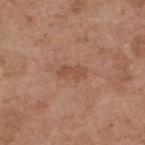Q: Was a biopsy performed?
A: no biopsy performed (imaged during a skin exam)
Q: What kind of image is this?
A: ~15 mm crop, total-body skin-cancer survey
Q: Where on the body is the lesion?
A: the upper back
Q: Patient demographics?
A: male, about 55 years old
Q: Lesion size?
A: ~3 mm (longest diameter)
Q: What did automated image analysis measure?
A: an eccentricity of roughly 0.9 and a shape-asymmetry score of about 0.3 (0 = symmetric); border irregularity of about 3.5 on a 0–10 scale and a color-variation rating of about 1.5/10
Q: What lighting was used for the tile?
A: white-light illumination